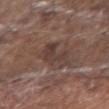No biopsy was performed on this lesion — it was imaged during a full skin examination and was not determined to be concerning.
On the right forearm.
The tile uses white-light illumination.
The patient is a male roughly 70 years of age.
A roughly 15 mm field-of-view crop from a total-body skin photograph.
Longest diameter approximately 6 mm.